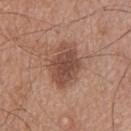biopsy_status: not biopsied; imaged during a skin examination
patient:
  sex: male
  age_approx: 65
site: upper back
lesion_size:
  long_diameter_mm_approx: 5.5
lighting: white-light
image:
  source: total-body photography crop
  field_of_view_mm: 15